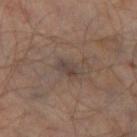Clinical impression:
This lesion was catalogued during total-body skin photography and was not selected for biopsy.
Context:
A lesion tile, about 15 mm wide, cut from a 3D total-body photograph. The lesion is on the left thigh. Longest diameter approximately 2.5 mm. A male subject, roughly 65 years of age. This is a cross-polarized tile.